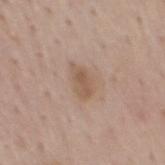Clinical impression: The lesion was photographed on a routine skin check and not biopsied; there is no pathology result. Image and clinical context: Located on the mid back. This is a white-light tile. Cropped from a total-body skin-imaging series; the visible field is about 15 mm. The patient is a male aged approximately 55. Measured at roughly 3.5 mm in maximum diameter.